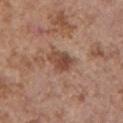– notes: total-body-photography surveillance lesion; no biopsy
– diameter: ≈3.5 mm
– automated lesion analysis: an average lesion color of about L≈47 a*≈21 b*≈28 (CIELAB) and roughly 11 lightness units darker than nearby skin; a border-irregularity rating of about 2.5/10, a color-variation rating of about 4.5/10, and a peripheral color-asymmetry measure near 1.5; a nevus-likeness score of about 80/100 and a lesion-detection confidence of about 100/100
– image: ~15 mm crop, total-body skin-cancer survey
– tile lighting: white-light illumination
– site: the left upper arm
– subject: female, in their mid-60s